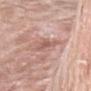Impression: This lesion was catalogued during total-body skin photography and was not selected for biopsy. Clinical summary: On the chest. This is a white-light tile. A male subject, about 60 years old. The recorded lesion diameter is about 2.5 mm. A lesion tile, about 15 mm wide, cut from a 3D total-body photograph.